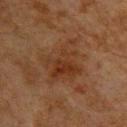follow-up = total-body-photography surveillance lesion; no biopsy
tile lighting = cross-polarized illumination
patient = male, aged 63–67
imaging modality = ~15 mm tile from a whole-body skin photo
lesion diameter = ≈6.5 mm
anatomic site = the upper back